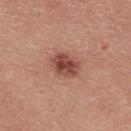follow-up: catalogued during a skin exam; not biopsied
location: the upper back
image-analysis metrics: a footprint of about 8 mm², a shape eccentricity near 0.7, and a shape-asymmetry score of about 0.15 (0 = symmetric); a lesion color around L≈47 a*≈26 b*≈27 in CIELAB and a normalized lesion–skin contrast near 9.5; a color-variation rating of about 4.5/10 and a peripheral color-asymmetry measure near 1.5
diameter: about 3.5 mm
acquisition: 15 mm crop, total-body photography
subject: male, in their 40s